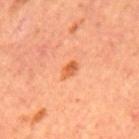Clinical impression:
The lesion was tiled from a total-body skin photograph and was not biopsied.
Image and clinical context:
A roughly 15 mm field-of-view crop from a total-body skin photograph. The subject is a male aged around 65. From the mid back.